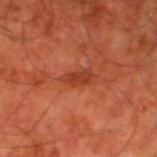biopsy status: catalogued during a skin exam; not biopsied
diameter: ~3 mm (longest diameter)
image-analysis metrics: a footprint of about 4 mm² and an outline eccentricity of about 0.9 (0 = round, 1 = elongated); a lesion color around L≈31 a*≈27 b*≈29 in CIELAB, a lesion–skin lightness drop of about 7, and a normalized lesion–skin contrast near 6.5
lighting: cross-polarized illumination
image: ~15 mm crop, total-body skin-cancer survey
site: the left lower leg
subject: male, about 80 years old